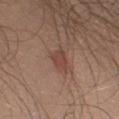biopsy status: total-body-photography surveillance lesion; no biopsy